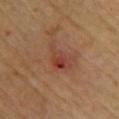{"biopsy_status": "not biopsied; imaged during a skin examination", "site": "back", "lesion_size": {"long_diameter_mm_approx": 3.5}, "image": {"source": "total-body photography crop", "field_of_view_mm": 15}, "automated_metrics": {"nevus_likeness_0_100": 0}, "lighting": "cross-polarized", "patient": {"sex": "female", "age_approx": 65}}